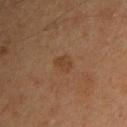<case>
<biopsy_status>not biopsied; imaged during a skin examination</biopsy_status>
<image>
  <source>total-body photography crop</source>
  <field_of_view_mm>15</field_of_view_mm>
</image>
<site>arm</site>
<lesion_size>
  <long_diameter_mm_approx>2.5</long_diameter_mm_approx>
</lesion_size>
<patient>
  <sex>male</sex>
  <age_approx>45</age_approx>
</patient>
<lighting>cross-polarized</lighting>
</case>